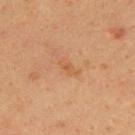Clinical impression:
Imaged during a routine full-body skin examination; the lesion was not biopsied and no histopathology is available.
Acquisition and patient details:
An algorithmic analysis of the crop reported a footprint of about 2 mm², an eccentricity of roughly 0.9, and a symmetry-axis asymmetry near 0.35. The analysis additionally found an average lesion color of about L≈49 a*≈21 b*≈35 (CIELAB), about 5 CIELAB-L* units darker than the surrounding skin, and a normalized lesion–skin contrast near 5. It also reported internal color variation of about 0 on a 0–10 scale. The subject is a female in their 40s. Located on the upper back. Imaged with cross-polarized lighting. A lesion tile, about 15 mm wide, cut from a 3D total-body photograph.